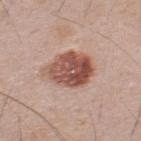biopsy status = total-body-photography surveillance lesion; no biopsy
subject = male, in their mid- to late 30s
image = total-body-photography crop, ~15 mm field of view
body site = the upper back
diameter = ≈5 mm
TBP lesion metrics = a lesion area of about 16 mm², a shape eccentricity near 0.5, and a symmetry-axis asymmetry near 0.15; border irregularity of about 1.5 on a 0–10 scale, a color-variation rating of about 7/10, and radial color variation of about 2.5